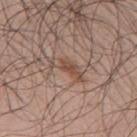| key | value |
|---|---|
| notes | catalogued during a skin exam; not biopsied |
| patient | male, aged around 50 |
| site | the upper back |
| imaging modality | total-body-photography crop, ~15 mm field of view |